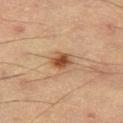Assessment: Part of a total-body skin-imaging series; this lesion was reviewed on a skin check and was not flagged for biopsy.